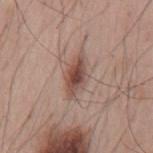<record>
<biopsy_status>not biopsied; imaged during a skin examination</biopsy_status>
<patient>
  <sex>male</sex>
  <age_approx>55</age_approx>
</patient>
<image>
  <source>total-body photography crop</source>
  <field_of_view_mm>15</field_of_view_mm>
</image>
<lesion_size>
  <long_diameter_mm_approx>5.0</long_diameter_mm_approx>
</lesion_size>
<site>mid back</site>
<automated_metrics>
  <area_mm2_approx>8.0</area_mm2_approx>
  <shape_asymmetry>0.15</shape_asymmetry>
  <cielab_L>49</cielab_L>
  <cielab_a>19</cielab_a>
  <cielab_b>24</cielab_b>
  <vs_skin_darker_L>13.0</vs_skin_darker_L>
  <nevus_likeness_0_100>95</nevus_likeness_0_100>
  <lesion_detection_confidence_0_100>100</lesion_detection_confidence_0_100>
</automated_metrics>
</record>